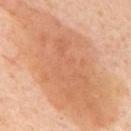Q: Is there a histopathology result?
A: imaged on a skin check; not biopsied
Q: What is the anatomic site?
A: the back
Q: What are the patient's age and sex?
A: male, aged around 65
Q: What is the imaging modality?
A: total-body-photography crop, ~15 mm field of view
Q: How was the tile lit?
A: cross-polarized illumination